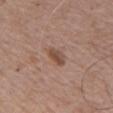{
  "biopsy_status": "not biopsied; imaged during a skin examination",
  "patient": {
    "sex": "male",
    "age_approx": 65
  },
  "site": "chest",
  "automated_metrics": {
    "vs_skin_darker_L": 10.0,
    "color_variation_0_10": 3.5,
    "peripheral_color_asymmetry": 1.0,
    "nevus_likeness_0_100": 55,
    "lesion_detection_confidence_0_100": 100
  },
  "image": {
    "source": "total-body photography crop",
    "field_of_view_mm": 15
  },
  "lesion_size": {
    "long_diameter_mm_approx": 3.0
  }
}